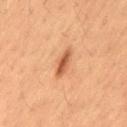notes — total-body-photography surveillance lesion; no biopsy
body site — the left thigh
image-analysis metrics — an average lesion color of about L≈53 a*≈25 b*≈36 (CIELAB), about 12 CIELAB-L* units darker than the surrounding skin, and a normalized border contrast of about 8; a border-irregularity index near 3.5/10, internal color variation of about 2.5 on a 0–10 scale, and peripheral color asymmetry of about 1; a classifier nevus-likeness of about 85/100 and lesion-presence confidence of about 100/100
patient — male, in their mid- to late 30s
lesion diameter — ≈4 mm
tile lighting — cross-polarized
imaging modality — total-body-photography crop, ~15 mm field of view